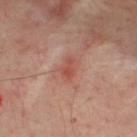Assessment:
This lesion was catalogued during total-body skin photography and was not selected for biopsy.
Image and clinical context:
Automated image analysis of the tile measured a mean CIELAB color near L≈49 a*≈27 b*≈29, a lesion–skin lightness drop of about 8, and a normalized border contrast of about 6.5. It also reported a border-irregularity rating of about 3.5/10, a within-lesion color-variation index near 1.5/10, and radial color variation of about 0.5. The analysis additionally found a nevus-likeness score of about 0/100. This is a cross-polarized tile. The lesion's longest dimension is about 2.5 mm. The patient is a male about 50 years old. The lesion is located on the upper back. This image is a 15 mm lesion crop taken from a total-body photograph.